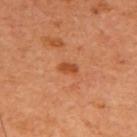Captured under cross-polarized illumination.
A female patient.
Located on the upper back.
Automated tile analysis of the lesion measured an automated nevus-likeness rating near 70 out of 100 and a detector confidence of about 100 out of 100 that the crop contains a lesion.
This image is a 15 mm lesion crop taken from a total-body photograph.
Approximately 2 mm at its widest.